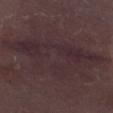biopsy status=no biopsy performed (imaged during a skin exam); size=~13 mm (longest diameter); image=total-body-photography crop, ~15 mm field of view; subject=female, roughly 30 years of age; automated lesion analysis=roughly 5 lightness units darker than nearby skin; location=the right thigh.